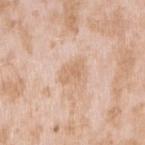<tbp_lesion>
<biopsy_status>not biopsied; imaged during a skin examination</biopsy_status>
<patient>
  <sex>female</sex>
  <age_approx>25</age_approx>
</patient>
<site>arm</site>
<lighting>white-light</lighting>
<image>
  <source>total-body photography crop</source>
  <field_of_view_mm>15</field_of_view_mm>
</image>
<lesion_size>
  <long_diameter_mm_approx>3.0</long_diameter_mm_approx>
</lesion_size>
<automated_metrics>
  <cielab_L>67</cielab_L>
  <cielab_a>18</cielab_a>
  <cielab_b>33</cielab_b>
  <nevus_likeness_0_100>0</nevus_likeness_0_100>
  <lesion_detection_confidence_0_100>100</lesion_detection_confidence_0_100>
</automated_metrics>
</tbp_lesion>